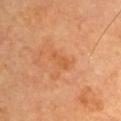On the left upper arm.
Longest diameter approximately 2.5 mm.
A male subject roughly 70 years of age.
A lesion tile, about 15 mm wide, cut from a 3D total-body photograph.
The tile uses cross-polarized illumination.